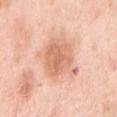Q: Automated lesion metrics?
A: a border-irregularity rating of about 4.5/10 and a color-variation rating of about 4/10; a classifier nevus-likeness of about 5/100 and a detector confidence of about 100 out of 100 that the crop contains a lesion
Q: How large is the lesion?
A: ≈7 mm
Q: Lesion location?
A: the right upper arm
Q: What are the patient's age and sex?
A: female, aged 63 to 67
Q: What is the imaging modality?
A: total-body-photography crop, ~15 mm field of view